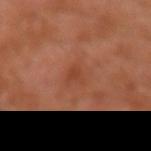- workup: no biopsy performed (imaged during a skin exam)
- illumination: cross-polarized illumination
- image-analysis metrics: a lesion area of about 3 mm², an eccentricity of roughly 0.75, and two-axis asymmetry of about 0.45
- subject: male, approximately 30 years of age
- body site: the left upper arm
- acquisition: total-body-photography crop, ~15 mm field of view
- size: ~2.5 mm (longest diameter)